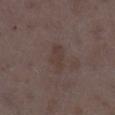biopsy status — no biopsy performed (imaged during a skin exam); acquisition — total-body-photography crop, ~15 mm field of view; subject — female, aged around 35; site — the right thigh.